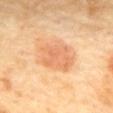<tbp_lesion>
<biopsy_status>not biopsied; imaged during a skin examination</biopsy_status>
<lighting>cross-polarized</lighting>
<patient>
  <sex>female</sex>
  <age_approx>60</age_approx>
</patient>
<automated_metrics>
  <border_irregularity_0_10>2.5</border_irregularity_0_10>
  <color_variation_0_10>3.0</color_variation_0_10>
  <peripheral_color_asymmetry>1.0</peripheral_color_asymmetry>
  <nevus_likeness_0_100>65</nevus_likeness_0_100>
  <lesion_detection_confidence_0_100>100</lesion_detection_confidence_0_100>
</automated_metrics>
<site>mid back</site>
<image>
  <source>total-body photography crop</source>
  <field_of_view_mm>15</field_of_view_mm>
</image>
<lesion_size>
  <long_diameter_mm_approx>5.0</long_diameter_mm_approx>
</lesion_size>
</tbp_lesion>